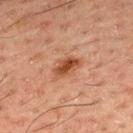No biopsy was performed on this lesion — it was imaged during a full skin examination and was not determined to be concerning.
The lesion's longest dimension is about 3.5 mm.
On the upper back.
An algorithmic analysis of the crop reported an area of roughly 5.5 mm², an outline eccentricity of about 0.85 (0 = round, 1 = elongated), and two-axis asymmetry of about 0.25. The analysis additionally found lesion-presence confidence of about 100/100.
A male patient, aged approximately 50.
A close-up tile cropped from a whole-body skin photograph, about 15 mm across.
The tile uses cross-polarized illumination.